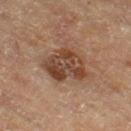  biopsy_status: not biopsied; imaged during a skin examination
  image:
    source: total-body photography crop
    field_of_view_mm: 15
  automated_metrics:
    area_mm2_approx: 16.0
    eccentricity: 0.4
    shape_asymmetry: 0.3
    cielab_L: 37
    cielab_a: 17
    cielab_b: 25
    vs_skin_contrast_norm: 8.5
    border_irregularity_0_10: 3.0
    color_variation_0_10: 5.5
    peripheral_color_asymmetry: 1.5
    nevus_likeness_0_100: 15
    lesion_detection_confidence_0_100: 100
  lesion_size:
    long_diameter_mm_approx: 5.0
  site: right thigh
  patient:
    sex: female
    age_approx: 55
  lighting: cross-polarized